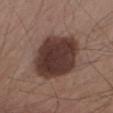A close-up tile cropped from a whole-body skin photograph, about 15 mm across. From the right upper arm. A male subject about 55 years old. Approximately 8.5 mm at its widest. Automated image analysis of the tile measured an area of roughly 31 mm², an outline eccentricity of about 0.85 (0 = round, 1 = elongated), and a shape-asymmetry score of about 0.15 (0 = symmetric). The analysis additionally found a normalized lesion–skin contrast near 13. And it measured a border-irregularity index near 2/10, a color-variation rating of about 4.5/10, and radial color variation of about 1.5. The analysis additionally found lesion-presence confidence of about 100/100.